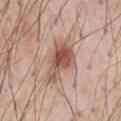The lesion was tiled from a total-body skin photograph and was not biopsied. Cropped from a whole-body photographic skin survey; the tile spans about 15 mm. On the front of the torso. A male subject, in their mid-50s. The tile uses white-light illumination. Longest diameter approximately 5.5 mm.